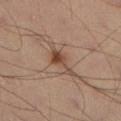Assessment: Part of a total-body skin-imaging series; this lesion was reviewed on a skin check and was not flagged for biopsy. Clinical summary: Automated image analysis of the tile measured an average lesion color of about L≈45 a*≈18 b*≈27 (CIELAB), about 10 CIELAB-L* units darker than the surrounding skin, and a normalized border contrast of about 8. A 15 mm crop from a total-body photograph taken for skin-cancer surveillance. From the right lower leg. A male subject, aged approximately 40. Imaged with cross-polarized lighting. Approximately 4 mm at its widest.